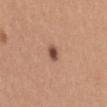<lesion>
  <biopsy_status>not biopsied; imaged during a skin examination</biopsy_status>
  <lesion_size>
    <long_diameter_mm_approx>2.5</long_diameter_mm_approx>
  </lesion_size>
  <patient>
    <sex>female</sex>
    <age_approx>35</age_approx>
  </patient>
  <image>
    <source>total-body photography crop</source>
    <field_of_view_mm>15</field_of_view_mm>
  </image>
  <lighting>white-light</lighting>
  <site>mid back</site>
</lesion>